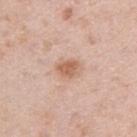Imaged during a routine full-body skin examination; the lesion was not biopsied and no histopathology is available. The recorded lesion diameter is about 2.5 mm. The lesion is on the right upper arm. A roughly 15 mm field-of-view crop from a total-body skin photograph. Captured under white-light illumination. Automated image analysis of the tile measured a lesion color around L≈61 a*≈21 b*≈32 in CIELAB and roughly 11 lightness units darker than nearby skin. A male subject, about 40 years old.